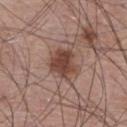Impression: Imaged during a routine full-body skin examination; the lesion was not biopsied and no histopathology is available. Image and clinical context: Approximately 4 mm at its widest. The lesion is located on the right lower leg. A male subject, aged 58 to 62. Captured under white-light illumination. A region of skin cropped from a whole-body photographic capture, roughly 15 mm wide.